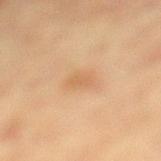Imaged during a routine full-body skin examination; the lesion was not biopsied and no histopathology is available. A region of skin cropped from a whole-body photographic capture, roughly 15 mm wide. An algorithmic analysis of the crop reported a footprint of about 4 mm², an eccentricity of roughly 0.8, and a symmetry-axis asymmetry near 0.3. The software also gave border irregularity of about 2.5 on a 0–10 scale, a within-lesion color-variation index near 1.5/10, and peripheral color asymmetry of about 0.5. The lesion is on the left lower leg. A female patient, in their mid- to late 50s. The lesion's longest dimension is about 3 mm. Imaged with cross-polarized lighting.